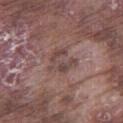Part of a total-body skin-imaging series; this lesion was reviewed on a skin check and was not flagged for biopsy. This image is a 15 mm lesion crop taken from a total-body photograph. From the right thigh. Longest diameter approximately 3.5 mm. Captured under white-light illumination. The subject is a male aged around 75.